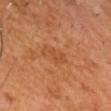  biopsy_status: not biopsied; imaged during a skin examination
  site: head or neck
  patient:
    sex: male
    age_approx: 60
  lesion_size:
    long_diameter_mm_approx: 3.5
  image:
    source: total-body photography crop
    field_of_view_mm: 15
  lighting: cross-polarized